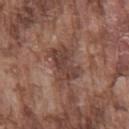Imaged during a routine full-body skin examination; the lesion was not biopsied and no histopathology is available. The lesion is on the chest. A male subject, in their mid-70s. A 15 mm crop from a total-body photograph taken for skin-cancer surveillance.